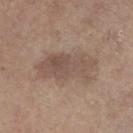Imaged during a routine full-body skin examination; the lesion was not biopsied and no histopathology is available. On the leg. The subject is a female approximately 65 years of age. A 15 mm close-up extracted from a 3D total-body photography capture.